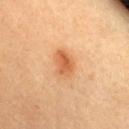{"site": "chest", "lighting": "cross-polarized", "image": {"source": "total-body photography crop", "field_of_view_mm": 15}, "patient": {"sex": "female", "age_approx": 30}, "automated_metrics": {"border_irregularity_0_10": 1.5, "lesion_detection_confidence_0_100": 100}}